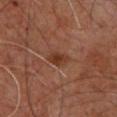<lesion>
<biopsy_status>not biopsied; imaged during a skin examination</biopsy_status>
<lighting>cross-polarized</lighting>
<site>upper back</site>
<image>
  <source>total-body photography crop</source>
  <field_of_view_mm>15</field_of_view_mm>
</image>
<patient>
  <sex>male</sex>
  <age_approx>60</age_approx>
</patient>
<lesion_size>
  <long_diameter_mm_approx>2.5</long_diameter_mm_approx>
</lesion_size>
<automated_metrics>
  <area_mm2_approx>4.0</area_mm2_approx>
  <eccentricity>0.75</eccentricity>
  <shape_asymmetry>0.25</shape_asymmetry>
  <nevus_likeness_0_100>85</nevus_likeness_0_100>
  <lesion_detection_confidence_0_100>100</lesion_detection_confidence_0_100>
</automated_metrics>
</lesion>